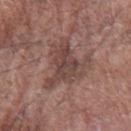Assessment: Captured during whole-body skin photography for melanoma surveillance; the lesion was not biopsied. Image and clinical context: A roughly 15 mm field-of-view crop from a total-body skin photograph. Longest diameter approximately 7 mm. An algorithmic analysis of the crop reported a border-irregularity rating of about 8.5/10, a within-lesion color-variation index near 4/10, and a peripheral color-asymmetry measure near 1. The tile uses white-light illumination. A male subject, in their mid- to late 70s. From the left forearm.